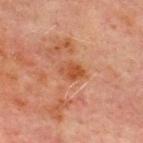Findings:
* follow-up · imaged on a skin check; not biopsied
* lighting · cross-polarized illumination
* anatomic site · the chest
* subject · male, about 70 years old
* imaging modality · total-body-photography crop, ~15 mm field of view